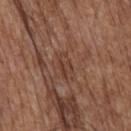The lesion was tiled from a total-body skin photograph and was not biopsied.
Approximately 3 mm at its widest.
A 15 mm crop from a total-body photograph taken for skin-cancer surveillance.
A male subject, aged approximately 75.
The lesion is located on the mid back.
Captured under white-light illumination.